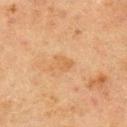<tbp_lesion>
  <biopsy_status>not biopsied; imaged during a skin examination</biopsy_status>
  <patient>
    <sex>male</sex>
    <age_approx>70</age_approx>
  </patient>
  <image>
    <source>total-body photography crop</source>
    <field_of_view_mm>15</field_of_view_mm>
  </image>
  <lesion_size>
    <long_diameter_mm_approx>2.5</long_diameter_mm_approx>
  </lesion_size>
  <site>back</site>
</tbp_lesion>